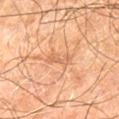The lesion-visualizer software estimated an outline eccentricity of about 0.9 (0 = round, 1 = elongated) and a symmetry-axis asymmetry near 0.35. The analysis additionally found an automated nevus-likeness rating near 0 out of 100 and a detector confidence of about 90 out of 100 that the crop contains a lesion. A close-up tile cropped from a whole-body skin photograph, about 15 mm across. The lesion is on the right leg. A male subject, about 60 years old. About 3.5 mm across. This is a cross-polarized tile.